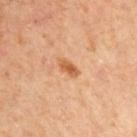| feature | finding |
|---|---|
| workup | catalogued during a skin exam; not biopsied |
| imaging modality | ~15 mm tile from a whole-body skin photo |
| patient | male, approximately 60 years of age |
| diameter | ≈3 mm |
| location | the right upper arm |